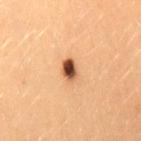follow-up — total-body-photography surveillance lesion; no biopsy | tile lighting — cross-polarized illumination | location — the right thigh | subject — female, about 25 years old | lesion size — ~3 mm (longest diameter) | imaging modality — ~15 mm crop, total-body skin-cancer survey.